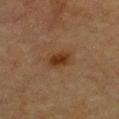The lesion was photographed on a routine skin check and not biopsied; there is no pathology result.
The total-body-photography lesion software estimated a footprint of about 5 mm², a shape eccentricity near 0.8, and two-axis asymmetry of about 0.25. And it measured a border-irregularity rating of about 2/10 and a within-lesion color-variation index near 2/10.
Located on the chest.
A roughly 15 mm field-of-view crop from a total-body skin photograph.
The patient is a male aged 83 or over.
About 3 mm across.